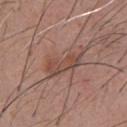Recorded during total-body skin imaging; not selected for excision or biopsy.
An algorithmic analysis of the crop reported a lesion color around L≈48 a*≈20 b*≈25 in CIELAB, about 8 CIELAB-L* units darker than the surrounding skin, and a normalized border contrast of about 6. The software also gave an automated nevus-likeness rating near 10 out of 100 and a lesion-detection confidence of about 100/100.
The lesion is located on the chest.
Imaged with white-light lighting.
Approximately 5 mm at its widest.
The subject is a male roughly 55 years of age.
A close-up tile cropped from a whole-body skin photograph, about 15 mm across.